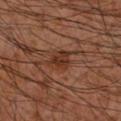{
  "biopsy_status": "not biopsied; imaged during a skin examination",
  "image": {
    "source": "total-body photography crop",
    "field_of_view_mm": 15
  },
  "patient": {
    "sex": "male",
    "age_approx": 55
  },
  "lesion_size": {
    "long_diameter_mm_approx": 3.0
  },
  "lighting": "cross-polarized",
  "site": "right forearm"
}